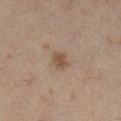follow-up: imaged on a skin check; not biopsied | image: 15 mm crop, total-body photography | patient: female, aged 38 to 42 | body site: the left leg.